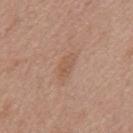<lesion>
<biopsy_status>not biopsied; imaged during a skin examination</biopsy_status>
<image>
  <source>total-body photography crop</source>
  <field_of_view_mm>15</field_of_view_mm>
</image>
<site>mid back</site>
<lesion_size>
  <long_diameter_mm_approx>3.5</long_diameter_mm_approx>
</lesion_size>
<patient>
  <sex>female</sex>
  <age_approx>75</age_approx>
</patient>
</lesion>